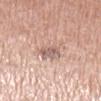The lesion was photographed on a routine skin check and not biopsied; there is no pathology result. Automated image analysis of the tile measured a lesion color around L≈63 a*≈18 b*≈24 in CIELAB, a lesion–skin lightness drop of about 9, and a lesion-to-skin contrast of about 5.5 (normalized; higher = more distinct). The software also gave a nevus-likeness score of about 0/100. Imaged with white-light lighting. On the left upper arm. Cropped from a total-body skin-imaging series; the visible field is about 15 mm. The patient is a female aged approximately 55.